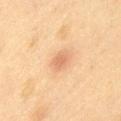Imaged during a routine full-body skin examination; the lesion was not biopsied and no histopathology is available.
Located on the left thigh.
A 15 mm close-up tile from a total-body photography series done for melanoma screening.
The lesion's longest dimension is about 2.5 mm.
A female subject, aged 58–62.
Imaged with cross-polarized lighting.